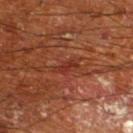| field | value |
|---|---|
| workup | catalogued during a skin exam; not biopsied |
| TBP lesion metrics | a footprint of about 3 mm², an outline eccentricity of about 0.9 (0 = round, 1 = elongated), and a shape-asymmetry score of about 0.3 (0 = symmetric); a border-irregularity index near 3.5/10; an automated nevus-likeness rating near 0 out of 100 and lesion-presence confidence of about 75/100 |
| image source | total-body-photography crop, ~15 mm field of view |
| location | the leg |
| illumination | cross-polarized illumination |
| size | ~2.5 mm (longest diameter) |
| patient | male, in their mid-60s |